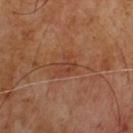imaging modality = ~15 mm tile from a whole-body skin photo | subject = male, in their mid- to late 60s | lesion size = ≈3 mm | lighting = cross-polarized illumination.